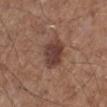Q: Was a biopsy performed?
A: no biopsy performed (imaged during a skin exam)
Q: What is the anatomic site?
A: the left lower leg
Q: What lighting was used for the tile?
A: white-light illumination
Q: What did automated image analysis measure?
A: a mean CIELAB color near L≈40 a*≈19 b*≈24, about 11 CIELAB-L* units darker than the surrounding skin, and a normalized lesion–skin contrast near 9; a border-irregularity index near 1.5/10, a color-variation rating of about 3.5/10, and a peripheral color-asymmetry measure near 1
Q: How large is the lesion?
A: about 4 mm
Q: What kind of image is this?
A: ~15 mm crop, total-body skin-cancer survey
Q: Who is the patient?
A: male, aged around 60